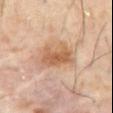{
  "biopsy_status": "not biopsied; imaged during a skin examination",
  "lesion_size": {
    "long_diameter_mm_approx": 4.5
  },
  "patient": {
    "sex": "male",
    "age_approx": 60
  },
  "image": {
    "source": "total-body photography crop",
    "field_of_view_mm": 15
  },
  "lighting": "cross-polarized",
  "site": "abdomen",
  "automated_metrics": {
    "vs_skin_darker_L": 10.0,
    "vs_skin_contrast_norm": 7.5,
    "nevus_likeness_0_100": 75,
    "lesion_detection_confidence_0_100": 100
  }
}